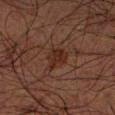| key | value |
|---|---|
| notes | no biopsy performed (imaged during a skin exam) |
| body site | the arm |
| lesion diameter | about 3 mm |
| automated lesion analysis | a border-irregularity rating of about 3/10, a within-lesion color-variation index near 2.5/10, and a peripheral color-asymmetry measure near 1 |
| image | 15 mm crop, total-body photography |
| patient | male, aged 68–72 |
| lighting | cross-polarized |